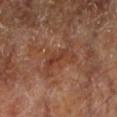workup: total-body-photography surveillance lesion; no biopsy | patient: about 65 years old | location: the left lower leg | image source: ~15 mm crop, total-body skin-cancer survey | illumination: cross-polarized illumination.